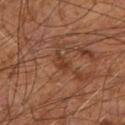| field | value |
|---|---|
| subject | male, approximately 60 years of age |
| acquisition | ~15 mm tile from a whole-body skin photo |
| lesion diameter | about 2.5 mm |
| lighting | cross-polarized illumination |
| location | the right leg |
| automated lesion analysis | a footprint of about 3 mm², a shape eccentricity near 0.85, and a symmetry-axis asymmetry near 0.55; a within-lesion color-variation index near 0/10 and radial color variation of about 0; an automated nevus-likeness rating near 0 out of 100 and a lesion-detection confidence of about 100/100 |